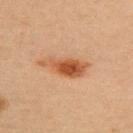Q: Was a biopsy performed?
A: catalogued during a skin exam; not biopsied
Q: What did automated image analysis measure?
A: a border-irregularity rating of about 4/10 and a within-lesion color-variation index near 5.5/10
Q: Lesion location?
A: the upper back
Q: What kind of image is this?
A: total-body-photography crop, ~15 mm field of view
Q: What is the lesion's diameter?
A: ~5.5 mm (longest diameter)
Q: Who is the patient?
A: female, in their mid- to late 40s